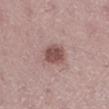The lesion is on the leg.
This image is a 15 mm lesion crop taken from a total-body photograph.
Imaged with white-light lighting.
The total-body-photography lesion software estimated a lesion area of about 6.5 mm², a shape eccentricity near 0.55, and a symmetry-axis asymmetry near 0.15.
A female subject aged 38–42.
Approximately 3 mm at its widest.